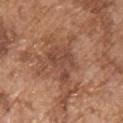No biopsy was performed on this lesion — it was imaged during a full skin examination and was not determined to be concerning.
A male subject aged around 75.
Automated tile analysis of the lesion measured an eccentricity of roughly 0.7.
Captured under white-light illumination.
A 15 mm close-up extracted from a 3D total-body photography capture.
On the chest.